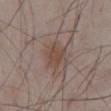A 15 mm close-up tile from a total-body photography series done for melanoma screening. The lesion is on the front of the torso. Captured under white-light illumination. The lesion-visualizer software estimated a lesion area of about 8 mm², a shape eccentricity near 0.6, and two-axis asymmetry of about 0.25. And it measured a mean CIELAB color near L≈46 a*≈15 b*≈24, roughly 7 lightness units darker than nearby skin, and a lesion-to-skin contrast of about 6.5 (normalized; higher = more distinct). And it measured an automated nevus-likeness rating near 45 out of 100 and a lesion-detection confidence of about 100/100. A male subject, aged 63–67.